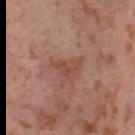Captured during whole-body skin photography for melanoma surveillance; the lesion was not biopsied. Automated image analysis of the tile measured a footprint of about 3.5 mm² and two-axis asymmetry of about 0.6. It also reported a border-irregularity index near 7/10, a within-lesion color-variation index near 0/10, and a peripheral color-asymmetry measure near 0. And it measured lesion-presence confidence of about 100/100. A female subject about 55 years old. Imaged with cross-polarized lighting. This image is a 15 mm lesion crop taken from a total-body photograph. The lesion is located on the leg.